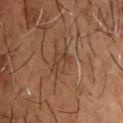This lesion was catalogued during total-body skin photography and was not selected for biopsy.